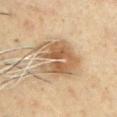Recorded during total-body skin imaging; not selected for excision or biopsy. The tile uses cross-polarized illumination. A region of skin cropped from a whole-body photographic capture, roughly 15 mm wide. From the left upper arm. The lesion's longest dimension is about 6 mm. A male subject, aged approximately 50.